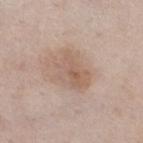Q: Who is the patient?
A: male, about 60 years old
Q: What is the anatomic site?
A: the right thigh
Q: What is the imaging modality?
A: ~15 mm tile from a whole-body skin photo
Q: How was the tile lit?
A: white-light